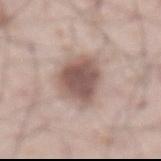Clinical impression: Part of a total-body skin-imaging series; this lesion was reviewed on a skin check and was not flagged for biopsy. Acquisition and patient details: Captured under white-light illumination. A male patient in their mid- to late 60s. Measured at roughly 6 mm in maximum diameter. The lesion is on the abdomen. Cropped from a whole-body photographic skin survey; the tile spans about 15 mm.